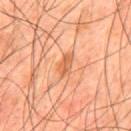Clinical impression:
No biopsy was performed on this lesion — it was imaged during a full skin examination and was not determined to be concerning.
Acquisition and patient details:
The lesion-visualizer software estimated a footprint of about 4 mm² and an eccentricity of roughly 0.85. On the mid back. Captured under cross-polarized illumination. A male patient, about 45 years old. Measured at roughly 3 mm in maximum diameter. A 15 mm close-up extracted from a 3D total-body photography capture.